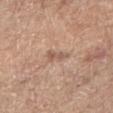Image and clinical context:
Longest diameter approximately 2.5 mm. Automated image analysis of the tile measured an area of roughly 3 mm², an eccentricity of roughly 0.85, and a shape-asymmetry score of about 0.4 (0 = symmetric). And it measured a normalized lesion–skin contrast near 6.5. A female subject aged approximately 75. A region of skin cropped from a whole-body photographic capture, roughly 15 mm wide. On the arm. Captured under white-light illumination.